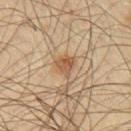Clinical impression: The lesion was photographed on a routine skin check and not biopsied; there is no pathology result. Background: Automated tile analysis of the lesion measured a lesion–skin lightness drop of about 10 and a normalized border contrast of about 7. It also reported an automated nevus-likeness rating near 85 out of 100 and a lesion-detection confidence of about 100/100. The recorded lesion diameter is about 2.5 mm. Imaged with cross-polarized lighting. The lesion is on the front of the torso. A 15 mm close-up extracted from a 3D total-body photography capture. The patient is a male about 60 years old.